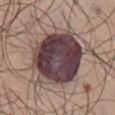Captured during whole-body skin photography for melanoma surveillance; the lesion was not biopsied.
A male subject aged around 75.
Located on the abdomen.
A roughly 15 mm field-of-view crop from a total-body skin photograph.
This is a white-light tile.
An algorithmic analysis of the crop reported a lesion area of about 36 mm², a shape eccentricity near 0.5, and a symmetry-axis asymmetry near 0.15. It also reported lesion-presence confidence of about 90/100.